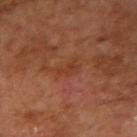Imaged with cross-polarized lighting.
A lesion tile, about 15 mm wide, cut from a 3D total-body photograph.
The lesion is on the right upper arm.
The patient is a male aged around 55.